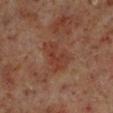Assessment:
Recorded during total-body skin imaging; not selected for excision or biopsy.